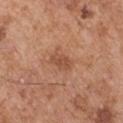  biopsy_status: not biopsied; imaged during a skin examination
  patient:
    sex: male
    age_approx: 55
  image:
    source: total-body photography crop
    field_of_view_mm: 15
  site: arm
  lesion_size:
    long_diameter_mm_approx: 2.5